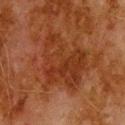Part of a total-body skin-imaging series; this lesion was reviewed on a skin check and was not flagged for biopsy. A male subject aged 78–82. On the upper back. Measured at roughly 7 mm in maximum diameter. A close-up tile cropped from a whole-body skin photograph, about 15 mm across.